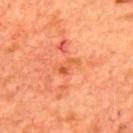Part of a total-body skin-imaging series; this lesion was reviewed on a skin check and was not flagged for biopsy.
Longest diameter approximately 3 mm.
The subject is a male aged around 70.
Located on the upper back.
Captured under cross-polarized illumination.
A 15 mm crop from a total-body photograph taken for skin-cancer surveillance.